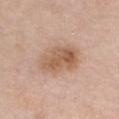The lesion was photographed on a routine skin check and not biopsied; there is no pathology result.